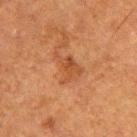biopsy status: imaged on a skin check; not biopsied
image-analysis metrics: a mean CIELAB color near L≈40 a*≈22 b*≈32, about 7 CIELAB-L* units darker than the surrounding skin, and a lesion-to-skin contrast of about 6 (normalized; higher = more distinct); a classifier nevus-likeness of about 0/100
lesion size: ~4 mm (longest diameter)
body site: the upper back
imaging modality: 15 mm crop, total-body photography
subject: male, about 50 years old
tile lighting: cross-polarized illumination